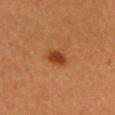Image and clinical context:
The lesion's longest dimension is about 3 mm. The subject is a female aged 28–32. From the front of the torso. Imaged with cross-polarized lighting. A 15 mm close-up tile from a total-body photography series done for melanoma screening.